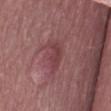This lesion was catalogued during total-body skin photography and was not selected for biopsy. A close-up tile cropped from a whole-body skin photograph, about 15 mm across. A female patient aged approximately 65. On the right thigh.